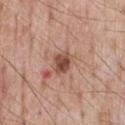  biopsy_status: not biopsied; imaged during a skin examination
  patient:
    sex: male
    age_approx: 55
  lighting: white-light
  site: chest
  image:
    source: total-body photography crop
    field_of_view_mm: 15
  lesion_size:
    long_diameter_mm_approx: 3.0